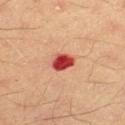Clinical impression:
The lesion was tiled from a total-body skin photograph and was not biopsied.